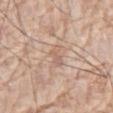No biopsy was performed on this lesion — it was imaged during a full skin examination and was not determined to be concerning.
The total-body-photography lesion software estimated an area of roughly 3.5 mm² and a shape eccentricity near 0.85. The analysis additionally found a border-irregularity rating of about 4/10, a color-variation rating of about 0.5/10, and peripheral color asymmetry of about 0. The analysis additionally found a detector confidence of about 90 out of 100 that the crop contains a lesion.
A male subject, aged approximately 80.
Located on the abdomen.
Cropped from a total-body skin-imaging series; the visible field is about 15 mm.
This is a white-light tile.